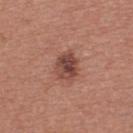biopsy status: imaged on a skin check; not biopsied
illumination: white-light illumination
anatomic site: the upper back
automated metrics: an automated nevus-likeness rating near 25 out of 100 and a detector confidence of about 100 out of 100 that the crop contains a lesion
diameter: about 3.5 mm
imaging modality: ~15 mm tile from a whole-body skin photo
subject: male, roughly 40 years of age